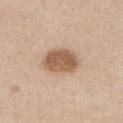No biopsy was performed on this lesion — it was imaged during a full skin examination and was not determined to be concerning. The lesion-visualizer software estimated a footprint of about 11 mm² and two-axis asymmetry of about 0.15. It also reported a border-irregularity rating of about 1.5/10, a color-variation rating of about 3.5/10, and radial color variation of about 1. The software also gave a classifier nevus-likeness of about 95/100 and a lesion-detection confidence of about 100/100. A female subject, aged 38–42. From the front of the torso. A 15 mm crop from a total-body photograph taken for skin-cancer surveillance. Approximately 4.5 mm at its widest.